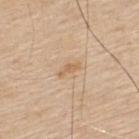Case summary:
- biopsy status — no biopsy performed (imaged during a skin exam)
- image-analysis metrics — a footprint of about 2.5 mm² and a symmetry-axis asymmetry near 0.45; internal color variation of about 0 on a 0–10 scale and peripheral color asymmetry of about 0; a nevus-likeness score of about 0/100 and lesion-presence confidence of about 100/100
- subject — male, aged around 80
- image source — 15 mm crop, total-body photography
- lighting — white-light
- size — about 3 mm
- location — the upper back